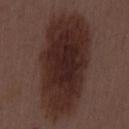<tbp_lesion>
<biopsy_status>not biopsied; imaged during a skin examination</biopsy_status>
<image>
  <source>total-body photography crop</source>
  <field_of_view_mm>15</field_of_view_mm>
</image>
<lighting>white-light</lighting>
<lesion_size>
  <long_diameter_mm_approx>13.5</long_diameter_mm_approx>
</lesion_size>
<patient>
  <sex>male</sex>
  <age_approx>55</age_approx>
</patient>
<site>mid back</site>
<automated_metrics>
  <vs_skin_darker_L>11.0</vs_skin_darker_L>
  <vs_skin_contrast_norm>11.0</vs_skin_contrast_norm>
  <lesion_detection_confidence_0_100>100</lesion_detection_confidence_0_100>
</automated_metrics>
</tbp_lesion>